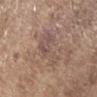{
  "site": "left forearm",
  "patient": {
    "sex": "male",
    "age_approx": 70
  },
  "image": {
    "source": "total-body photography crop",
    "field_of_view_mm": 15
  }
}